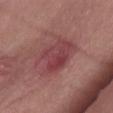The lesion was tiled from a total-body skin photograph and was not biopsied. A male patient, aged 53 to 57. An algorithmic analysis of the crop reported a classifier nevus-likeness of about 5/100 and a lesion-detection confidence of about 100/100. Captured under white-light illumination. This image is a 15 mm lesion crop taken from a total-body photograph. From the abdomen. The recorded lesion diameter is about 5.5 mm.